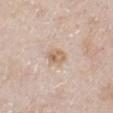Clinical impression: No biopsy was performed on this lesion — it was imaged during a full skin examination and was not determined to be concerning. Clinical summary: A male subject, aged 78–82. This image is a 15 mm lesion crop taken from a total-body photograph. The lesion's longest dimension is about 3 mm. The lesion is on the left lower leg. The tile uses white-light illumination.